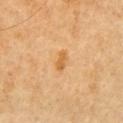workup: imaged on a skin check; not biopsied
image source: 15 mm crop, total-body photography
subject: female, aged approximately 50
TBP lesion metrics: a lesion area of about 3.5 mm², an outline eccentricity of about 0.85 (0 = round, 1 = elongated), and two-axis asymmetry of about 0.25; a classifier nevus-likeness of about 35/100 and lesion-presence confidence of about 100/100
tile lighting: cross-polarized
location: the arm